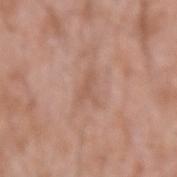Imaged during a routine full-body skin examination; the lesion was not biopsied and no histopathology is available.
This is a white-light tile.
The subject is a male aged 58–62.
The total-body-photography lesion software estimated an eccentricity of roughly 0.85. The analysis additionally found border irregularity of about 6.5 on a 0–10 scale, internal color variation of about 0 on a 0–10 scale, and a peripheral color-asymmetry measure near 0.
Approximately 3 mm at its widest.
Located on the left forearm.
Cropped from a whole-body photographic skin survey; the tile spans about 15 mm.